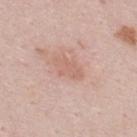Q: Was this lesion biopsied?
A: total-body-photography surveillance lesion; no biopsy
Q: What is the anatomic site?
A: the upper back
Q: What is the imaging modality?
A: total-body-photography crop, ~15 mm field of view
Q: Who is the patient?
A: male, aged approximately 25